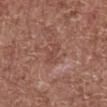{
  "biopsy_status": "not biopsied; imaged during a skin examination",
  "image": {
    "source": "total-body photography crop",
    "field_of_view_mm": 15
  },
  "lesion_size": {
    "long_diameter_mm_approx": 2.5
  },
  "lighting": "white-light",
  "patient": {
    "sex": "male",
    "age_approx": 65
  },
  "site": "abdomen"
}